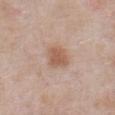notes: imaged on a skin check; not biopsied | lighting: white-light | site: the abdomen | image: 15 mm crop, total-body photography | image-analysis metrics: a mean CIELAB color near L≈57 a*≈19 b*≈30, about 10 CIELAB-L* units darker than the surrounding skin, and a normalized border contrast of about 7.5 | lesion diameter: ≈3 mm | patient: male, approximately 55 years of age.